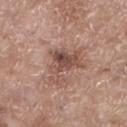No biopsy was performed on this lesion — it was imaged during a full skin examination and was not determined to be concerning. The recorded lesion diameter is about 6 mm. A female patient, aged 73–77. This is a white-light tile. The lesion is on the leg. A lesion tile, about 15 mm wide, cut from a 3D total-body photograph.